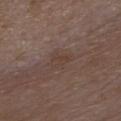<case>
<biopsy_status>not biopsied; imaged during a skin examination</biopsy_status>
<patient>
  <sex>male</sex>
  <age_approx>75</age_approx>
</patient>
<site>front of the torso</site>
<image>
  <source>total-body photography crop</source>
  <field_of_view_mm>15</field_of_view_mm>
</image>
<automated_metrics>
  <color_variation_0_10>1.5</color_variation_0_10>
  <nevus_likeness_0_100>0</nevus_likeness_0_100>
  <lesion_detection_confidence_0_100>100</lesion_detection_confidence_0_100>
</automated_metrics>
<lesion_size>
  <long_diameter_mm_approx>3.0</long_diameter_mm_approx>
</lesion_size>
</case>